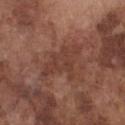{
  "biopsy_status": "not biopsied; imaged during a skin examination",
  "site": "chest",
  "lighting": "white-light",
  "patient": {
    "sex": "male",
    "age_approx": 75
  },
  "image": {
    "source": "total-body photography crop",
    "field_of_view_mm": 15
  }
}